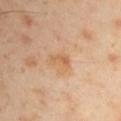biopsy status=total-body-photography surveillance lesion; no biopsy | subject=male, aged around 45 | size=~2.5 mm (longest diameter) | illumination=cross-polarized illumination | automated metrics=a footprint of about 3.5 mm², an eccentricity of roughly 0.8, and two-axis asymmetry of about 0.4; a nevus-likeness score of about 0/100 and a lesion-detection confidence of about 100/100 | body site=the arm | acquisition=total-body-photography crop, ~15 mm field of view.